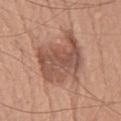follow-up = total-body-photography surveillance lesion; no biopsy | tile lighting = white-light | anatomic site = the chest | image = 15 mm crop, total-body photography | size = ≈7 mm | automated metrics = a border-irregularity index near 5/10, a color-variation rating of about 4/10, and a peripheral color-asymmetry measure near 1.5; a classifier nevus-likeness of about 25/100 and a lesion-detection confidence of about 100/100 | subject = male, aged around 60.